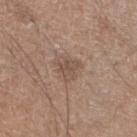The lesion was tiled from a total-body skin photograph and was not biopsied. This image is a 15 mm lesion crop taken from a total-body photograph. The patient is a male in their mid- to late 60s. From the right lower leg.